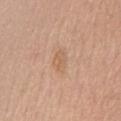follow-up = catalogued during a skin exam; not biopsied
image = 15 mm crop, total-body photography
patient = female, aged 53–57
illumination = white-light
TBP lesion metrics = a classifier nevus-likeness of about 0/100
size = about 2.5 mm
anatomic site = the front of the torso